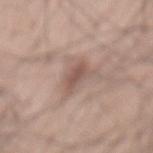Assessment: The lesion was tiled from a total-body skin photograph and was not biopsied.